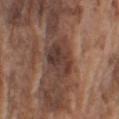{"biopsy_status": "not biopsied; imaged during a skin examination", "lighting": "white-light", "patient": {"sex": "male", "age_approx": 75}, "site": "arm", "lesion_size": {"long_diameter_mm_approx": 5.0}, "image": {"source": "total-body photography crop", "field_of_view_mm": 15}, "automated_metrics": {"area_mm2_approx": 14.0, "eccentricity": 0.7, "shape_asymmetry": 0.25, "vs_skin_darker_L": 10.0, "vs_skin_contrast_norm": 9.5}}